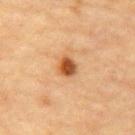Findings:
– workup — no biopsy performed (imaged during a skin exam)
– subject — male, aged around 85 or older
– acquisition — ~15 mm tile from a whole-body skin photo
– lighting — cross-polarized
– site — the chest